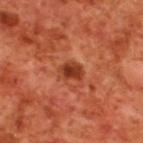workup: no biopsy performed (imaged during a skin exam); lighting: cross-polarized; size: ~2.5 mm (longest diameter); site: the upper back; imaging modality: 15 mm crop, total-body photography; TBP lesion metrics: a footprint of about 5 mm², a shape eccentricity near 0.65, and a symmetry-axis asymmetry near 0.35; patient: male, about 70 years old.